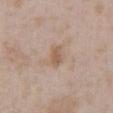{
  "image": {
    "source": "total-body photography crop",
    "field_of_view_mm": 15
  },
  "patient": {
    "sex": "male",
    "age_approx": 50
  },
  "lighting": "white-light",
  "automated_metrics": {
    "area_mm2_approx": 3.5,
    "eccentricity": 0.85,
    "shape_asymmetry": 0.25,
    "cielab_L": 57,
    "cielab_a": 17,
    "cielab_b": 29,
    "vs_skin_darker_L": 9.0,
    "vs_skin_contrast_norm": 6.5,
    "color_variation_0_10": 1.5,
    "peripheral_color_asymmetry": 0.5
  },
  "lesion_size": {
    "long_diameter_mm_approx": 2.5
  },
  "site": "abdomen"
}